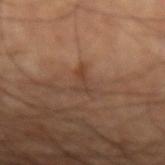This lesion was catalogued during total-body skin photography and was not selected for biopsy. The lesion is on the left forearm. Cropped from a total-body skin-imaging series; the visible field is about 15 mm. About 3 mm across. This is a cross-polarized tile. A male patient aged 58 to 62. An algorithmic analysis of the crop reported a mean CIELAB color near L≈39 a*≈18 b*≈27, roughly 6 lightness units darker than nearby skin, and a normalized lesion–skin contrast near 5.5. It also reported a border-irregularity index near 7.5/10, a within-lesion color-variation index near 0/10, and a peripheral color-asymmetry measure near 0.